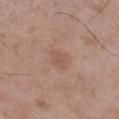Findings:
– follow-up: catalogued during a skin exam; not biopsied
– subject: male, aged approximately 50
– body site: the right lower leg
– imaging modality: ~15 mm tile from a whole-body skin photo